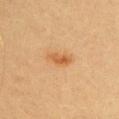- workup: imaged on a skin check; not biopsied
- location: the chest
- tile lighting: cross-polarized
- acquisition: 15 mm crop, total-body photography
- TBP lesion metrics: an average lesion color of about L≈52 a*≈22 b*≈38 (CIELAB), a lesion–skin lightness drop of about 9, and a normalized lesion–skin contrast near 7; a classifier nevus-likeness of about 90/100 and a lesion-detection confidence of about 100/100
- size: about 3 mm
- patient: female, in their 20s